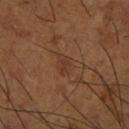Recorded during total-body skin imaging; not selected for excision or biopsy.
This image is a 15 mm lesion crop taken from a total-body photograph.
From the right lower leg.
Longest diameter approximately 2.5 mm.
Automated image analysis of the tile measured an area of roughly 3.5 mm² and a shape-asymmetry score of about 0.3 (0 = symmetric). It also reported a mean CIELAB color near L≈35 a*≈20 b*≈28, roughly 5 lightness units darker than nearby skin, and a normalized border contrast of about 5. It also reported a nevus-likeness score of about 0/100.
A male subject in their mid-60s.